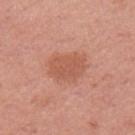Part of a total-body skin-imaging series; this lesion was reviewed on a skin check and was not flagged for biopsy. The lesion-visualizer software estimated an outline eccentricity of about 0.65 (0 = round, 1 = elongated) and a shape-asymmetry score of about 0.2 (0 = symmetric). A female patient, aged 48–52. The lesion is located on the left upper arm. Imaged with white-light lighting. A 15 mm close-up extracted from a 3D total-body photography capture. The lesion's longest dimension is about 4 mm.